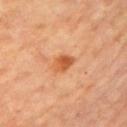Assessment:
The lesion was photographed on a routine skin check and not biopsied; there is no pathology result.
Context:
This is a cross-polarized tile. Measured at roughly 3 mm in maximum diameter. A female patient roughly 65 years of age. From the left upper arm. This image is a 15 mm lesion crop taken from a total-body photograph.